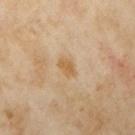workup: imaged on a skin check; not biopsied
illumination: cross-polarized
image source: 15 mm crop, total-body photography
subject: female, about 35 years old
diameter: ≈2.5 mm
anatomic site: the arm